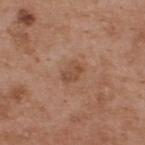Q: Was this lesion biopsied?
A: catalogued during a skin exam; not biopsied
Q: Who is the patient?
A: male, about 55 years old
Q: What kind of image is this?
A: ~15 mm crop, total-body skin-cancer survey
Q: Where on the body is the lesion?
A: the upper back